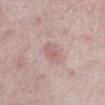| field | value |
|---|---|
| workup | total-body-photography surveillance lesion; no biopsy |
| patient | female, in their mid-40s |
| image-analysis metrics | border irregularity of about 1.5 on a 0–10 scale, a color-variation rating of about 2.5/10, and a peripheral color-asymmetry measure near 1; a nevus-likeness score of about 20/100 and a detector confidence of about 100 out of 100 that the crop contains a lesion |
| lesion diameter | about 2.5 mm |
| body site | the left lower leg |
| image | ~15 mm crop, total-body skin-cancer survey |